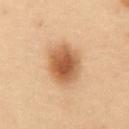The lesion's longest dimension is about 5 mm.
The tile uses cross-polarized illumination.
From the abdomen.
A region of skin cropped from a whole-body photographic capture, roughly 15 mm wide.
The patient is a male aged 53 to 57.
The lesion-visualizer software estimated a footprint of about 16 mm² and two-axis asymmetry of about 0.1. The software also gave an average lesion color of about L≈47 a*≈18 b*≈31 (CIELAB), roughly 12 lightness units darker than nearby skin, and a lesion-to-skin contrast of about 9 (normalized; higher = more distinct).